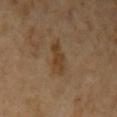Recorded during total-body skin imaging; not selected for excision or biopsy. A female subject, aged 68–72. The lesion-visualizer software estimated a lesion area of about 7.5 mm², an eccentricity of roughly 0.9, and a shape-asymmetry score of about 0.25 (0 = symmetric). And it measured an average lesion color of about L≈41 a*≈16 b*≈32 (CIELAB), a lesion–skin lightness drop of about 7, and a normalized border contrast of about 7. The recorded lesion diameter is about 4.5 mm. Located on the left upper arm. A 15 mm close-up extracted from a 3D total-body photography capture. The tile uses cross-polarized illumination.